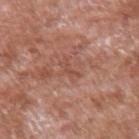A 15 mm close-up extracted from a 3D total-body photography capture.
A male patient aged approximately 60.
Located on the left upper arm.
The lesion-visualizer software estimated a footprint of about 2.5 mm², an eccentricity of roughly 0.9, and a symmetry-axis asymmetry near 0.55. The software also gave an average lesion color of about L≈50 a*≈24 b*≈28 (CIELAB), about 6 CIELAB-L* units darker than the surrounding skin, and a normalized lesion–skin contrast near 4.5. The software also gave a classifier nevus-likeness of about 0/100.
This is a white-light tile.